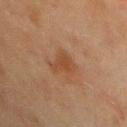| feature | finding |
|---|---|
| biopsy status | catalogued during a skin exam; not biopsied |
| automated metrics | a shape eccentricity near 0.8 and a symmetry-axis asymmetry near 0.4; a mean CIELAB color near L≈37 a*≈18 b*≈29 and a normalized lesion–skin contrast near 6; a border-irregularity rating of about 3.5/10 and a color-variation rating of about 1.5/10; an automated nevus-likeness rating near 5 out of 100 |
| subject | female, aged 48 to 52 |
| imaging modality | ~15 mm crop, total-body skin-cancer survey |
| body site | the chest |